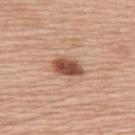Assessment:
The lesion was photographed on a routine skin check and not biopsied; there is no pathology result.
Image and clinical context:
A female subject, aged 63 to 67. Imaged with white-light lighting. On the upper back. A region of skin cropped from a whole-body photographic capture, roughly 15 mm wide.